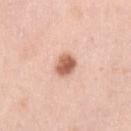The lesion was tiled from a total-body skin photograph and was not biopsied. This image is a 15 mm lesion crop taken from a total-body photograph. A female subject roughly 40 years of age. The lesion is located on the left upper arm.